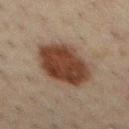Q: Is there a histopathology result?
A: total-body-photography surveillance lesion; no biopsy
Q: Patient demographics?
A: male, aged approximately 50
Q: What is the imaging modality?
A: ~15 mm crop, total-body skin-cancer survey
Q: Automated lesion metrics?
A: a classifier nevus-likeness of about 100/100 and a lesion-detection confidence of about 100/100
Q: Illumination type?
A: cross-polarized illumination
Q: What is the anatomic site?
A: the chest